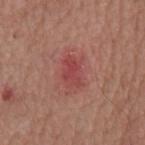Impression: The lesion was tiled from a total-body skin photograph and was not biopsied. Context: Imaged with white-light lighting. From the back. A region of skin cropped from a whole-body photographic capture, roughly 15 mm wide. Measured at roughly 3.5 mm in maximum diameter. An algorithmic analysis of the crop reported a lesion color around L≈46 a*≈31 b*≈24 in CIELAB and about 8 CIELAB-L* units darker than the surrounding skin. A male patient, roughly 65 years of age.